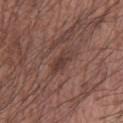Recorded during total-body skin imaging; not selected for excision or biopsy. A lesion tile, about 15 mm wide, cut from a 3D total-body photograph. Captured under white-light illumination. The lesion is located on the left forearm. The total-body-photography lesion software estimated a lesion area of about 4 mm², a shape eccentricity near 0.9, and a symmetry-axis asymmetry near 0.3. And it measured border irregularity of about 3.5 on a 0–10 scale and radial color variation of about 1. The analysis additionally found a classifier nevus-likeness of about 5/100. The lesion's longest dimension is about 3.5 mm. The subject is a male aged approximately 65.